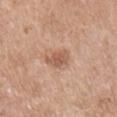Clinical impression:
The lesion was tiled from a total-body skin photograph and was not biopsied.
Acquisition and patient details:
A 15 mm crop from a total-body photograph taken for skin-cancer surveillance. The lesion is located on the right upper arm. A female patient, about 55 years old. The tile uses white-light illumination.